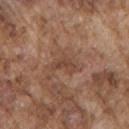– location — the arm
– subject — male, aged 73 to 77
– image source — total-body-photography crop, ~15 mm field of view
– illumination — white-light
– TBP lesion metrics — a border-irregularity index near 5.5/10 and internal color variation of about 1 on a 0–10 scale